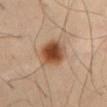The lesion was photographed on a routine skin check and not biopsied; there is no pathology result. A region of skin cropped from a whole-body photographic capture, roughly 15 mm wide. A male subject about 55 years old. The lesion-visualizer software estimated a lesion area of about 10 mm², a shape eccentricity near 0.65, and two-axis asymmetry of about 0.15. The analysis additionally found a lesion color around L≈46 a*≈21 b*≈32 in CIELAB, a lesion–skin lightness drop of about 15, and a normalized lesion–skin contrast near 11.5. Longest diameter approximately 4 mm. On the abdomen.